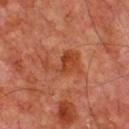The lesion was tiled from a total-body skin photograph and was not biopsied. This is a cross-polarized tile. A male patient, approximately 70 years of age. The lesion is located on the chest. About 5 mm across. A close-up tile cropped from a whole-body skin photograph, about 15 mm across.